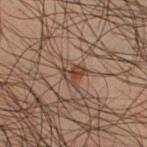Recorded during total-body skin imaging; not selected for excision or biopsy.